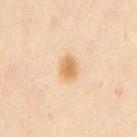body site: the chest; patient: male, aged 43 to 47; image source: total-body-photography crop, ~15 mm field of view.